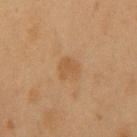Q: Is there a histopathology result?
A: imaged on a skin check; not biopsied
Q: What are the patient's age and sex?
A: male, aged approximately 55
Q: How was this image acquired?
A: total-body-photography crop, ~15 mm field of view
Q: Where on the body is the lesion?
A: the chest